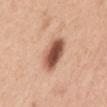workup — imaged on a skin check; not biopsied | body site — the front of the torso | subject — female, approximately 30 years of age | automated lesion analysis — a mean CIELAB color near L≈55 a*≈23 b*≈30 and about 18 CIELAB-L* units darker than the surrounding skin; a border-irregularity rating of about 2/10, a within-lesion color-variation index near 7.5/10, and a peripheral color-asymmetry measure near 2.5; an automated nevus-likeness rating near 100 out of 100 and lesion-presence confidence of about 100/100 | image — ~15 mm crop, total-body skin-cancer survey | illumination — white-light | size — ~4.5 mm (longest diameter).